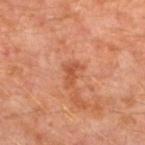<lesion>
<site>left lower leg</site>
<image>
  <source>total-body photography crop</source>
  <field_of_view_mm>15</field_of_view_mm>
</image>
<patient>
  <sex>male</sex>
  <age_approx>30</age_approx>
</patient>
</lesion>